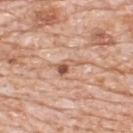notes: catalogued during a skin exam; not biopsied | body site: the upper back | image: 15 mm crop, total-body photography | subject: male, approximately 80 years of age | lighting: white-light illumination | diameter: ~3 mm (longest diameter) | automated metrics: a lesion area of about 3.5 mm² and an eccentricity of roughly 0.85; a lesion-to-skin contrast of about 7.5 (normalized; higher = more distinct); border irregularity of about 5.5 on a 0–10 scale, a within-lesion color-variation index near 4/10, and a peripheral color-asymmetry measure near 1.5.